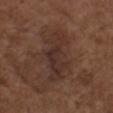The lesion was tiled from a total-body skin photograph and was not biopsied.